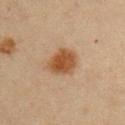Clinical impression: Part of a total-body skin-imaging series; this lesion was reviewed on a skin check and was not flagged for biopsy. Clinical summary: The lesion is located on the right upper arm. A 15 mm close-up extracted from a 3D total-body photography capture. The patient is a male about 60 years old. The lesion's longest dimension is about 4 mm.